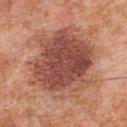Imaged during a routine full-body skin examination; the lesion was not biopsied and no histopathology is available. A 15 mm close-up tile from a total-body photography series done for melanoma screening. Longest diameter approximately 8.5 mm. Located on the chest. A male subject, in their mid-50s. The tile uses white-light illumination.